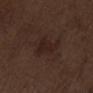Assessment:
Imaged during a routine full-body skin examination; the lesion was not biopsied and no histopathology is available.
Acquisition and patient details:
From the abdomen. Longest diameter approximately 4 mm. A close-up tile cropped from a whole-body skin photograph, about 15 mm across. The subject is a male aged 68 to 72. The tile uses white-light illumination.